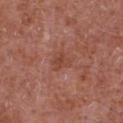Case summary:
• follow-up — catalogued during a skin exam; not biopsied
• body site — the chest
• image — total-body-photography crop, ~15 mm field of view
• patient — male, aged 63 to 67